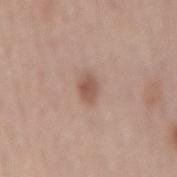Assessment:
Recorded during total-body skin imaging; not selected for excision or biopsy.
Clinical summary:
A close-up tile cropped from a whole-body skin photograph, about 15 mm across. Automated image analysis of the tile measured an average lesion color of about L≈54 a*≈20 b*≈26 (CIELAB) and a lesion-to-skin contrast of about 7.5 (normalized; higher = more distinct). The tile uses white-light illumination. A female subject, aged 63–67. Located on the mid back.